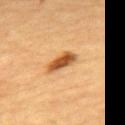<tbp_lesion>
<biopsy_status>not biopsied; imaged during a skin examination</biopsy_status>
<image>
  <source>total-body photography crop</source>
  <field_of_view_mm>15</field_of_view_mm>
</image>
<patient>
  <sex>male</sex>
  <age_approx>85+</age_approx>
</patient>
<site>upper back</site>
</tbp_lesion>